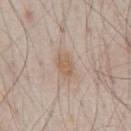No biopsy was performed on this lesion — it was imaged during a full skin examination and was not determined to be concerning.
A male subject, roughly 80 years of age.
A 15 mm close-up extracted from a 3D total-body photography capture.
The lesion is on the chest.